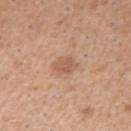Q: Was this lesion biopsied?
A: imaged on a skin check; not biopsied
Q: What is the anatomic site?
A: the right upper arm
Q: How was the tile lit?
A: white-light
Q: Patient demographics?
A: male, aged around 50
Q: Lesion size?
A: ~2.5 mm (longest diameter)
Q: How was this image acquired?
A: total-body-photography crop, ~15 mm field of view